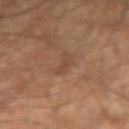Q: Was this lesion biopsied?
A: imaged on a skin check; not biopsied
Q: How was this image acquired?
A: total-body-photography crop, ~15 mm field of view
Q: Where on the body is the lesion?
A: the right upper arm
Q: What is the lesion's diameter?
A: ~3 mm (longest diameter)
Q: What are the patient's age and sex?
A: male, aged approximately 65